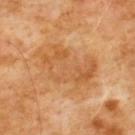image = total-body-photography crop, ~15 mm field of view
location = the chest
patient = male, aged 58 to 62
automated metrics = an average lesion color of about L≈55 a*≈22 b*≈40 (CIELAB); a border-irregularity index near 5.5/10 and a color-variation rating of about 4.5/10; a classifier nevus-likeness of about 0/100 and a detector confidence of about 100 out of 100 that the crop contains a lesion
lesion diameter = ≈7.5 mm
lighting = cross-polarized illumination